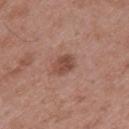Q: Was a biopsy performed?
A: catalogued during a skin exam; not biopsied
Q: Lesion size?
A: ~2.5 mm (longest diameter)
Q: Where on the body is the lesion?
A: the mid back
Q: How was this image acquired?
A: ~15 mm tile from a whole-body skin photo
Q: How was the tile lit?
A: white-light illumination
Q: Who is the patient?
A: male, approximately 55 years of age